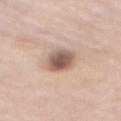Clinical impression: Part of a total-body skin-imaging series; this lesion was reviewed on a skin check and was not flagged for biopsy. Clinical summary: The lesion is located on the mid back. A close-up tile cropped from a whole-body skin photograph, about 15 mm across. A male patient, approximately 85 years of age or older. Imaged with white-light lighting. Automated image analysis of the tile measured a shape eccentricity near 0.7. The analysis additionally found border irregularity of about 1 on a 0–10 scale and peripheral color asymmetry of about 1.5. It also reported a lesion-detection confidence of about 100/100. The lesion's longest dimension is about 3.5 mm.